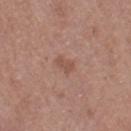Q: Was a biopsy performed?
A: total-body-photography surveillance lesion; no biopsy